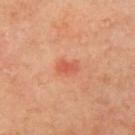{"biopsy_status": "not biopsied; imaged during a skin examination", "lighting": "cross-polarized", "image": {"source": "total-body photography crop", "field_of_view_mm": 15}, "site": "left upper arm", "automated_metrics": {"eccentricity": 0.8, "shape_asymmetry": 0.3, "cielab_L": 56, "cielab_a": 32, "cielab_b": 35, "vs_skin_darker_L": 8.0, "vs_skin_contrast_norm": 6.0, "border_irregularity_0_10": 3.0, "color_variation_0_10": 1.0, "peripheral_color_asymmetry": 0.5, "nevus_likeness_0_100": 5, "lesion_detection_confidence_0_100": 100}, "patient": {"sex": "male", "age_approx": 65}}